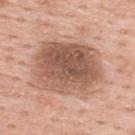Clinical impression: Part of a total-body skin-imaging series; this lesion was reviewed on a skin check and was not flagged for biopsy. Acquisition and patient details: A male subject aged 58 to 62. Cropped from a whole-body photographic skin survey; the tile spans about 15 mm. Imaged with white-light lighting. From the upper back. About 8 mm across.